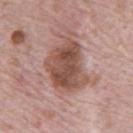<tbp_lesion>
<biopsy_status>not biopsied; imaged during a skin examination</biopsy_status>
<site>abdomen</site>
<image>
  <source>total-body photography crop</source>
  <field_of_view_mm>15</field_of_view_mm>
</image>
<patient>
  <sex>male</sex>
  <age_approx>70</age_approx>
</patient>
</tbp_lesion>